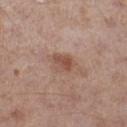The lesion was tiled from a total-body skin photograph and was not biopsied. Imaged with white-light lighting. From the leg. Measured at roughly 2.5 mm in maximum diameter. This image is a 15 mm lesion crop taken from a total-body photograph. A male patient, aged around 70.